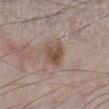Clinical impression: Captured during whole-body skin photography for melanoma surveillance; the lesion was not biopsied. Image and clinical context: The lesion is on the left lower leg. A close-up tile cropped from a whole-body skin photograph, about 15 mm across. The subject is a male about 70 years old.